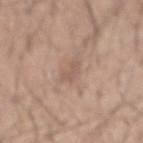Part of a total-body skin-imaging series; this lesion was reviewed on a skin check and was not flagged for biopsy. The lesion is located on the back. A male subject, aged 43–47. Cropped from a total-body skin-imaging series; the visible field is about 15 mm.